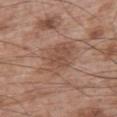{"biopsy_status": "not biopsied; imaged during a skin examination", "site": "left upper arm", "patient": {"sex": "male", "age_approx": 65}, "lesion_size": {"long_diameter_mm_approx": 5.5}, "image": {"source": "total-body photography crop", "field_of_view_mm": 15}}